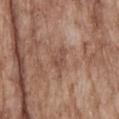The lesion was photographed on a routine skin check and not biopsied; there is no pathology result.
The subject is a male aged around 65.
The lesion's longest dimension is about 2.5 mm.
The total-body-photography lesion software estimated an average lesion color of about L≈49 a*≈20 b*≈26 (CIELAB). The software also gave lesion-presence confidence of about 100/100.
A lesion tile, about 15 mm wide, cut from a 3D total-body photograph.
The lesion is located on the head or neck.
The tile uses white-light illumination.